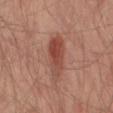Recorded during total-body skin imaging; not selected for excision or biopsy.
The lesion is on the left thigh.
Approximately 6 mm at its widest.
Captured under cross-polarized illumination.
A 15 mm close-up tile from a total-body photography series done for melanoma screening.
A male subject, aged around 65.